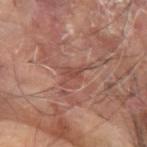Impression: Part of a total-body skin-imaging series; this lesion was reviewed on a skin check and was not flagged for biopsy. Background: A male patient aged 63 to 67. A 15 mm crop from a total-body photograph taken for skin-cancer surveillance. Approximately 3.5 mm at its widest. On the left forearm.